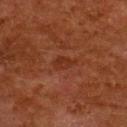automated metrics: a footprint of about 3.5 mm², a shape eccentricity near 0.85, and a shape-asymmetry score of about 0.25 (0 = symmetric); a classifier nevus-likeness of about 0/100 and a detector confidence of about 100 out of 100 that the crop contains a lesion | subject: female, aged 48 to 52 | lesion diameter: ~3 mm (longest diameter) | location: the upper back | image source: ~15 mm crop, total-body skin-cancer survey | lighting: cross-polarized illumination.